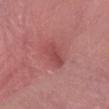A female subject, in their 60s. Located on the right forearm. The lesion's longest dimension is about 5 mm. The lesion-visualizer software estimated a lesion area of about 7.5 mm² and an eccentricity of roughly 0.9. It also reported a color-variation rating of about 3/10 and peripheral color asymmetry of about 1. The analysis additionally found an automated nevus-likeness rating near 0 out of 100 and a lesion-detection confidence of about 60/100. Cropped from a whole-body photographic skin survey; the tile spans about 15 mm.